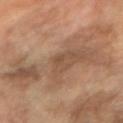No biopsy was performed on this lesion — it was imaged during a full skin examination and was not determined to be concerning. The lesion is located on the right forearm. A female patient approximately 70 years of age. A lesion tile, about 15 mm wide, cut from a 3D total-body photograph. The tile uses cross-polarized illumination.